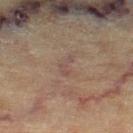Part of a total-body skin-imaging series; this lesion was reviewed on a skin check and was not flagged for biopsy.
The subject is a female aged around 80.
A roughly 15 mm field-of-view crop from a total-body skin photograph.
An algorithmic analysis of the crop reported an average lesion color of about L≈40 a*≈14 b*≈19 (CIELAB) and a normalized border contrast of about 5.5. The analysis additionally found a border-irregularity index near 7/10 and a color-variation rating of about 0/10.
Imaged with cross-polarized lighting.
The recorded lesion diameter is about 2.5 mm.
On the right thigh.